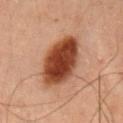Findings:
* biopsy status: total-body-photography surveillance lesion; no biopsy
* tile lighting: cross-polarized illumination
* patient: male, in their 50s
* imaging modality: ~15 mm tile from a whole-body skin photo
* lesion diameter: about 6.5 mm
* anatomic site: the abdomen